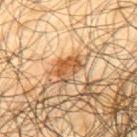follow-up: catalogued during a skin exam; not biopsied
size: ~3.5 mm (longest diameter)
body site: the back
image: ~15 mm tile from a whole-body skin photo
subject: male, aged 63 to 67
lighting: cross-polarized illumination